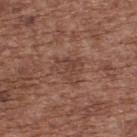No biopsy was performed on this lesion — it was imaged during a full skin examination and was not determined to be concerning. Measured at roughly 4 mm in maximum diameter. A male patient, in their mid-70s. A 15 mm close-up extracted from a 3D total-body photography capture. The lesion is on the back. This is a white-light tile.